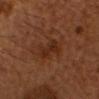A male subject about 55 years old.
On the head or neck.
The total-body-photography lesion software estimated a mean CIELAB color near L≈25 a*≈20 b*≈27, roughly 6 lightness units darker than nearby skin, and a normalized lesion–skin contrast near 6.5. It also reported border irregularity of about 4 on a 0–10 scale.
About 3 mm across.
This is a cross-polarized tile.
Cropped from a whole-body photographic skin survey; the tile spans about 15 mm.